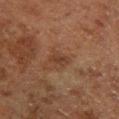{"biopsy_status": "not biopsied; imaged during a skin examination", "lesion_size": {"long_diameter_mm_approx": 2.5}, "image": {"source": "total-body photography crop", "field_of_view_mm": 15}, "patient": {"sex": "male", "age_approx": 80}, "lighting": "cross-polarized", "site": "left lower leg"}